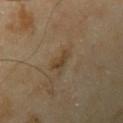Q: What lighting was used for the tile?
A: cross-polarized
Q: How was this image acquired?
A: ~15 mm tile from a whole-body skin photo
Q: What are the patient's age and sex?
A: male, about 65 years old
Q: What did automated image analysis measure?
A: an eccentricity of roughly 0.8 and a symmetry-axis asymmetry near 0.4; a lesion-to-skin contrast of about 7 (normalized; higher = more distinct); a border-irregularity index near 4/10; a classifier nevus-likeness of about 0/100 and a detector confidence of about 100 out of 100 that the crop contains a lesion
Q: Lesion location?
A: the left upper arm
Q: How large is the lesion?
A: about 2.5 mm